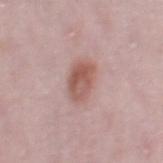Part of a total-body skin-imaging series; this lesion was reviewed on a skin check and was not flagged for biopsy. Located on the right thigh. The recorded lesion diameter is about 4 mm. A female subject, aged 28–32. The total-body-photography lesion software estimated an area of roughly 8.5 mm², a shape eccentricity near 0.75, and a symmetry-axis asymmetry near 0.15. The software also gave a normalized border contrast of about 8. And it measured an automated nevus-likeness rating near 85 out of 100 and a detector confidence of about 100 out of 100 that the crop contains a lesion. A lesion tile, about 15 mm wide, cut from a 3D total-body photograph.